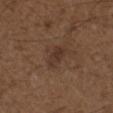Recorded during total-body skin imaging; not selected for excision or biopsy. Measured at roughly 3.5 mm in maximum diameter. Captured under white-light illumination. The subject is a male aged 48 to 52. On the front of the torso. A 15 mm close-up tile from a total-body photography series done for melanoma screening.